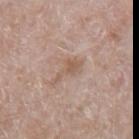biopsy_status: not biopsied; imaged during a skin examination
image:
  source: total-body photography crop
  field_of_view_mm: 15
site: arm
automated_metrics:
  area_mm2_approx: 4.0
  eccentricity: 0.9
  shape_asymmetry: 0.55
  cielab_L: 56
  cielab_a: 17
  cielab_b: 27
  vs_skin_darker_L: 8.0
patient:
  sex: female
  age_approx: 55